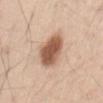Assessment:
Imaged during a routine full-body skin examination; the lesion was not biopsied and no histopathology is available.
Image and clinical context:
Automated image analysis of the tile measured a mean CIELAB color near L≈57 a*≈21 b*≈31 and about 17 CIELAB-L* units darker than the surrounding skin. The software also gave a border-irregularity index near 2/10 and a peripheral color-asymmetry measure near 1.5. The software also gave an automated nevus-likeness rating near 100 out of 100. Captured under white-light illumination. A male subject about 30 years old. The lesion's longest dimension is about 5.5 mm. The lesion is located on the back. Cropped from a whole-body photographic skin survey; the tile spans about 15 mm.